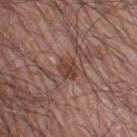Findings:
- workup: no biopsy performed (imaged during a skin exam)
- body site: the right thigh
- subject: male, roughly 60 years of age
- automated lesion analysis: an area of roughly 4.5 mm², an eccentricity of roughly 0.55, and two-axis asymmetry of about 0.15; a mean CIELAB color near L≈42 a*≈21 b*≈25, about 9 CIELAB-L* units darker than the surrounding skin, and a normalized border contrast of about 7.5
- illumination: white-light illumination
- size: ≈2.5 mm
- acquisition: total-body-photography crop, ~15 mm field of view